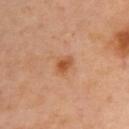Imaged with cross-polarized lighting. The lesion's longest dimension is about 2.5 mm. A female subject aged 38–42. Automated image analysis of the tile measured a footprint of about 4 mm², a shape eccentricity near 0.6, and two-axis asymmetry of about 0.25. The software also gave roughly 10 lightness units darker than nearby skin and a lesion-to-skin contrast of about 7.5 (normalized; higher = more distinct). And it measured a border-irregularity index near 2/10, a within-lesion color-variation index near 4/10, and peripheral color asymmetry of about 1. It also reported lesion-presence confidence of about 100/100. A roughly 15 mm field-of-view crop from a total-body skin photograph. The lesion is located on the upper back.